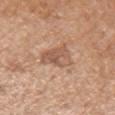The lesion was photographed on a routine skin check and not biopsied; there is no pathology result.
A male patient about 65 years old.
A 15 mm close-up extracted from a 3D total-body photography capture.
The lesion is on the arm.
This is a white-light tile.
Approximately 4 mm at its widest.